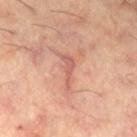Q: Was a biopsy performed?
A: imaged on a skin check; not biopsied
Q: How large is the lesion?
A: ≈4 mm
Q: How was this image acquired?
A: total-body-photography crop, ~15 mm field of view
Q: What is the anatomic site?
A: the right thigh
Q: What lighting was used for the tile?
A: cross-polarized
Q: Patient demographics?
A: male, about 60 years old